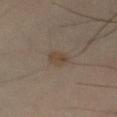Assessment: This lesion was catalogued during total-body skin photography and was not selected for biopsy. Acquisition and patient details: A male patient, roughly 40 years of age. The lesion is on the arm. A 15 mm close-up tile from a total-body photography series done for melanoma screening. Measured at roughly 2.5 mm in maximum diameter. This is a cross-polarized tile.